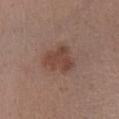Background: Automated tile analysis of the lesion measured an average lesion color of about L≈44 a*≈20 b*≈25 (CIELAB), a lesion–skin lightness drop of about 9, and a normalized border contrast of about 7. The analysis additionally found border irregularity of about 3.5 on a 0–10 scale, internal color variation of about 2.5 on a 0–10 scale, and a peripheral color-asymmetry measure near 1. The software also gave an automated nevus-likeness rating near 70 out of 100 and a detector confidence of about 100 out of 100 that the crop contains a lesion. From the right lower leg. This is a white-light tile. Longest diameter approximately 4.5 mm. A region of skin cropped from a whole-body photographic capture, roughly 15 mm wide. A male subject, roughly 55 years of age.